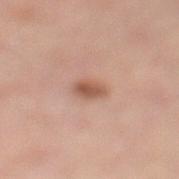Q: Is there a histopathology result?
A: imaged on a skin check; not biopsied
Q: How large is the lesion?
A: ~3 mm (longest diameter)
Q: How was this image acquired?
A: 15 mm crop, total-body photography
Q: Automated lesion metrics?
A: a lesion color around L≈53 a*≈21 b*≈28 in CIELAB, roughly 11 lightness units darker than nearby skin, and a lesion-to-skin contrast of about 8 (normalized; higher = more distinct)
Q: What lighting was used for the tile?
A: cross-polarized
Q: Where on the body is the lesion?
A: the left lower leg
Q: Who is the patient?
A: male, aged 53 to 57